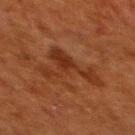automated_metrics:
  shape_asymmetry: 0.8
  border_irregularity_0_10: 10.0
  peripheral_color_asymmetry: 0.5
site: upper back
lighting: cross-polarized
image:
  source: total-body photography crop
  field_of_view_mm: 15
patient:
  sex: female
  age_approx: 50